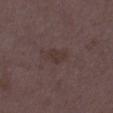No biopsy was performed on this lesion — it was imaged during a full skin examination and was not determined to be concerning.
About 2.5 mm across.
A female subject, aged approximately 35.
The total-body-photography lesion software estimated an area of roughly 4 mm², a shape eccentricity near 0.7, and a shape-asymmetry score of about 0.25 (0 = symmetric).
From the right thigh.
Cropped from a whole-body photographic skin survey; the tile spans about 15 mm.